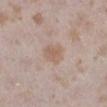The lesion was photographed on a routine skin check and not biopsied; there is no pathology result. From the right lower leg. The lesion-visualizer software estimated an eccentricity of roughly 0.65 and a shape-asymmetry score of about 0.2 (0 = symmetric). The software also gave border irregularity of about 2 on a 0–10 scale and internal color variation of about 1.5 on a 0–10 scale. The software also gave an automated nevus-likeness rating near 30 out of 100. Cropped from a whole-body photographic skin survey; the tile spans about 15 mm. The patient is a female roughly 25 years of age. About 3 mm across.